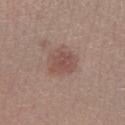biopsy status: catalogued during a skin exam; not biopsied | acquisition: total-body-photography crop, ~15 mm field of view | anatomic site: the leg | illumination: white-light | patient: male, in their mid- to late 40s | lesion diameter: ~3.5 mm (longest diameter) | image-analysis metrics: a lesion area of about 9.5 mm² and a shape eccentricity near 0.5; a border-irregularity index near 2/10, internal color variation of about 2.5 on a 0–10 scale, and peripheral color asymmetry of about 1.